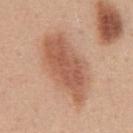Notes:
- biopsy status — no biopsy performed (imaged during a skin exam)
- subject — male, roughly 50 years of age
- imaging modality — ~15 mm crop, total-body skin-cancer survey
- body site — the chest
- lighting — white-light illumination
- diameter — about 8.5 mm